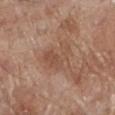notes = no biopsy performed (imaged during a skin exam); imaging modality = ~15 mm crop, total-body skin-cancer survey; subject = male, in their 70s; site = the left lower leg.